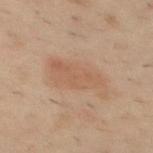{"biopsy_status": "not biopsied; imaged during a skin examination", "site": "upper back", "image": {"source": "total-body photography crop", "field_of_view_mm": 15}, "lighting": "cross-polarized", "patient": {"sex": "male", "age_approx": 40}, "lesion_size": {"long_diameter_mm_approx": 6.0}}